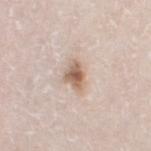* notes · imaged on a skin check; not biopsied
* image · ~15 mm tile from a whole-body skin photo
* TBP lesion metrics · a footprint of about 6 mm², an eccentricity of roughly 0.75, and a symmetry-axis asymmetry near 0.35; an average lesion color of about L≈62 a*≈16 b*≈28 (CIELAB) and about 13 CIELAB-L* units darker than the surrounding skin; a nevus-likeness score of about 100/100 and a lesion-detection confidence of about 100/100
* patient · male, aged 78–82
* location · the left thigh